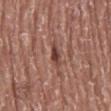location: the mid back | image: 15 mm crop, total-body photography | subject: male, in their mid- to late 70s | lighting: white-light illumination | automated metrics: a footprint of about 3 mm², an outline eccentricity of about 0.85 (0 = round, 1 = elongated), and two-axis asymmetry of about 0.35; roughly 12 lightness units darker than nearby skin and a lesion-to-skin contrast of about 9 (normalized; higher = more distinct); a classifier nevus-likeness of about 80/100 and a lesion-detection confidence of about 100/100.